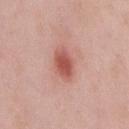notes: no biopsy performed (imaged during a skin exam)
TBP lesion metrics: an outline eccentricity of about 0.8 (0 = round, 1 = elongated) and a symmetry-axis asymmetry near 0.25; a border-irregularity index near 2.5/10, internal color variation of about 3 on a 0–10 scale, and radial color variation of about 0.5; an automated nevus-likeness rating near 100 out of 100 and a lesion-detection confidence of about 100/100
patient: male, aged approximately 55
body site: the chest
acquisition: 15 mm crop, total-body photography
tile lighting: white-light
lesion diameter: ≈4 mm